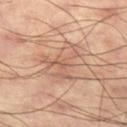site = the left thigh
acquisition = 15 mm crop, total-body photography
automated lesion analysis = an area of roughly 13 mm², a shape eccentricity near 0.75, and two-axis asymmetry of about 0.45; an average lesion color of about L≈58 a*≈20 b*≈30 (CIELAB) and roughly 7 lightness units darker than nearby skin; a color-variation rating of about 4.5/10 and a peripheral color-asymmetry measure near 1.5
diameter = ~5 mm (longest diameter)
lighting = cross-polarized illumination
patient = male, approximately 60 years of age